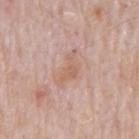{"biopsy_status": "not biopsied; imaged during a skin examination", "image": {"source": "total-body photography crop", "field_of_view_mm": 15}, "site": "mid back", "patient": {"sex": "male", "age_approx": 80}, "lesion_size": {"long_diameter_mm_approx": 4.5}, "automated_metrics": {"area_mm2_approx": 5.5, "eccentricity": 0.9, "shape_asymmetry": 0.65, "cielab_L": 61, "cielab_a": 20, "cielab_b": 28, "vs_skin_darker_L": 7.0, "vs_skin_contrast_norm": 6.0, "nevus_likeness_0_100": 0, "lesion_detection_confidence_0_100": 100}, "lighting": "white-light"}